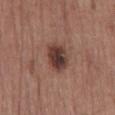{"biopsy_status": "not biopsied; imaged during a skin examination", "patient": {"sex": "male", "age_approx": 70}, "site": "front of the torso", "image": {"source": "total-body photography crop", "field_of_view_mm": 15}, "automated_metrics": {"area_mm2_approx": 9.0, "shape_asymmetry": 0.15, "nevus_likeness_0_100": 55, "lesion_detection_confidence_0_100": 100}}